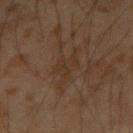notes: imaged on a skin check; not biopsied
location: the arm
imaging modality: total-body-photography crop, ~15 mm field of view
automated lesion analysis: an outline eccentricity of about 0.8 (0 = round, 1 = elongated) and a shape-asymmetry score of about 0.45 (0 = symmetric); an average lesion color of about L≈25 a*≈12 b*≈22 (CIELAB), a lesion–skin lightness drop of about 4, and a normalized lesion–skin contrast near 5
diameter: ≈5 mm
subject: male, aged 43–47
illumination: cross-polarized